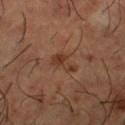follow-up: total-body-photography surveillance lesion; no biopsy | imaging modality: 15 mm crop, total-body photography | subject: male, approximately 65 years of age | location: the right thigh.